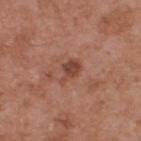Assessment:
This lesion was catalogued during total-body skin photography and was not selected for biopsy.
Context:
A male subject, aged around 55. The lesion is on the upper back. Automated image analysis of the tile measured a lesion area of about 4.5 mm², a shape eccentricity near 0.75, and two-axis asymmetry of about 0.4. It also reported an average lesion color of about L≈45 a*≈24 b*≈28 (CIELAB), roughly 10 lightness units darker than nearby skin, and a normalized border contrast of about 7.5. The software also gave a border-irregularity rating of about 4/10 and peripheral color asymmetry of about 0.5. And it measured a nevus-likeness score of about 25/100 and a detector confidence of about 100 out of 100 that the crop contains a lesion. A lesion tile, about 15 mm wide, cut from a 3D total-body photograph. The recorded lesion diameter is about 3 mm.